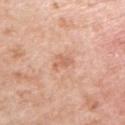This image is a 15 mm lesion crop taken from a total-body photograph. The lesion is on the right upper arm. This is a white-light tile. A female subject, roughly 70 years of age.